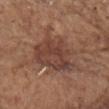biopsy_status: not biopsied; imaged during a skin examination
lighting: white-light
site: head or neck
image:
  source: total-body photography crop
  field_of_view_mm: 15
patient:
  sex: male
  age_approx: 80
lesion_size:
  long_diameter_mm_approx: 6.5